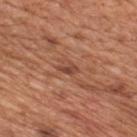Q: How was this image acquired?
A: ~15 mm crop, total-body skin-cancer survey
Q: What are the patient's age and sex?
A: male, aged approximately 65
Q: Automated lesion metrics?
A: a footprint of about 3 mm², an outline eccentricity of about 0.75 (0 = round, 1 = elongated), and a symmetry-axis asymmetry near 0.35; an average lesion color of about L≈46 a*≈24 b*≈30 (CIELAB), a lesion–skin lightness drop of about 9, and a normalized lesion–skin contrast near 6.5; a border-irregularity rating of about 3/10, a color-variation rating of about 3/10, and a peripheral color-asymmetry measure near 1
Q: What is the anatomic site?
A: the mid back